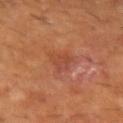The lesion was tiled from a total-body skin photograph and was not biopsied. An algorithmic analysis of the crop reported an area of roughly 6 mm², an outline eccentricity of about 0.6 (0 = round, 1 = elongated), and a symmetry-axis asymmetry near 0.35. It also reported an average lesion color of about L≈44 a*≈26 b*≈31 (CIELAB) and roughly 6 lightness units darker than nearby skin. The software also gave a color-variation rating of about 3.5/10 and a peripheral color-asymmetry measure near 1.5. The software also gave lesion-presence confidence of about 100/100. The recorded lesion diameter is about 3 mm. The tile uses cross-polarized illumination. The lesion is located on the left forearm. A 15 mm close-up tile from a total-body photography series done for melanoma screening. A male subject, in their 60s.